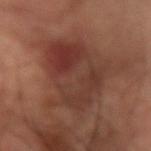biopsy status: total-body-photography surveillance lesion; no biopsy
image source: ~15 mm tile from a whole-body skin photo
body site: the arm
patient: male, in their 60s
size: about 8.5 mm
illumination: cross-polarized